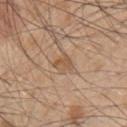Q: Was this lesion biopsied?
A: catalogued during a skin exam; not biopsied
Q: Lesion size?
A: ~2.5 mm (longest diameter)
Q: Illumination type?
A: white-light
Q: How was this image acquired?
A: 15 mm crop, total-body photography
Q: Patient demographics?
A: male, about 50 years old
Q: Automated lesion metrics?
A: a shape eccentricity near 0.8 and a symmetry-axis asymmetry near 0.3; a border-irregularity rating of about 3/10 and a within-lesion color-variation index near 1/10; a classifier nevus-likeness of about 5/100
Q: Lesion location?
A: the upper back